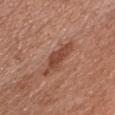notes: no biopsy performed (imaged during a skin exam); size: ~3.5 mm (longest diameter); anatomic site: the front of the torso; lighting: white-light; acquisition: total-body-photography crop, ~15 mm field of view; subject: female, approximately 65 years of age.